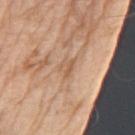No biopsy was performed on this lesion — it was imaged during a full skin examination and was not determined to be concerning. The patient is a male in their 70s. A 15 mm crop from a total-body photograph taken for skin-cancer surveillance. The lesion is on the arm. The lesion-visualizer software estimated an average lesion color of about L≈59 a*≈19 b*≈33 (CIELAB) and a lesion–skin lightness drop of about 8. About 2.5 mm across.